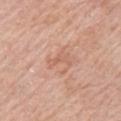Clinical impression:
No biopsy was performed on this lesion — it was imaged during a full skin examination and was not determined to be concerning.
Clinical summary:
This image is a 15 mm lesion crop taken from a total-body photograph. The lesion-visualizer software estimated an area of roughly 2.5 mm² and an eccentricity of roughly 0.9. The subject is a male roughly 80 years of age. Imaged with white-light lighting. About 3 mm across. From the chest.